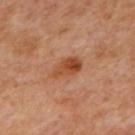notes=imaged on a skin check; not biopsied
illumination=cross-polarized
image=~15 mm tile from a whole-body skin photo
patient=in their mid-60s
size=about 4 mm
location=the mid back
TBP lesion metrics=a footprint of about 6.5 mm² and a symmetry-axis asymmetry near 0.25; a border-irregularity rating of about 2.5/10 and peripheral color asymmetry of about 2.5